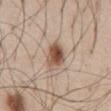Background: A male patient in their 60s. About 3.5 mm across. On the front of the torso. This is a white-light tile. This image is a 15 mm lesion crop taken from a total-body photograph. Automated image analysis of the tile measured an area of roughly 7.5 mm² and a symmetry-axis asymmetry near 0.25. And it measured border irregularity of about 2 on a 0–10 scale and a peripheral color-asymmetry measure near 2.5.